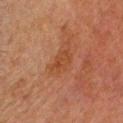Recorded during total-body skin imaging; not selected for excision or biopsy.
Longest diameter approximately 3 mm.
The tile uses cross-polarized illumination.
A lesion tile, about 15 mm wide, cut from a 3D total-body photograph.
Automated image analysis of the tile measured a mean CIELAB color near L≈37 a*≈21 b*≈30, about 5 CIELAB-L* units darker than the surrounding skin, and a normalized border contrast of about 5.5.
The lesion is on the head or neck.
A male patient in their 50s.